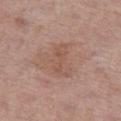Case summary:
- follow-up: catalogued during a skin exam; not biopsied
- automated lesion analysis: a lesion–skin lightness drop of about 6 and a normalized border contrast of about 5; border irregularity of about 5.5 on a 0–10 scale and peripheral color asymmetry of about 0.5; a classifier nevus-likeness of about 0/100 and lesion-presence confidence of about 100/100
- tile lighting: white-light illumination
- body site: the leg
- lesion size: ≈4.5 mm
- imaging modality: ~15 mm crop, total-body skin-cancer survey
- subject: female, aged approximately 60